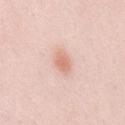This lesion was catalogued during total-body skin photography and was not selected for biopsy. Captured under white-light illumination. An algorithmic analysis of the crop reported a lesion area of about 4.5 mm², an outline eccentricity of about 0.7 (0 = round, 1 = elongated), and a symmetry-axis asymmetry near 0.2. And it measured an automated nevus-likeness rating near 100 out of 100. The lesion is located on the mid back. The lesion's longest dimension is about 3 mm. A 15 mm close-up extracted from a 3D total-body photography capture. The subject is a female about 15 years old.